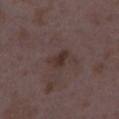Assessment:
The lesion was tiled from a total-body skin photograph and was not biopsied.
Clinical summary:
The total-body-photography lesion software estimated a footprint of about 4 mm², a shape eccentricity near 0.65, and a shape-asymmetry score of about 0.25 (0 = symmetric). The analysis additionally found a nevus-likeness score of about 25/100 and a lesion-detection confidence of about 100/100. A region of skin cropped from a whole-body photographic capture, roughly 15 mm wide. Imaged with white-light lighting. From the right thigh. The subject is a female aged 33–37.